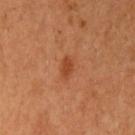No biopsy was performed on this lesion — it was imaged during a full skin examination and was not determined to be concerning. Measured at roughly 2.5 mm in maximum diameter. The lesion-visualizer software estimated a footprint of about 3 mm², an outline eccentricity of about 0.85 (0 = round, 1 = elongated), and a symmetry-axis asymmetry near 0.3. The software also gave a normalized lesion–skin contrast near 7. The software also gave a border-irregularity rating of about 2.5/10 and peripheral color asymmetry of about 0. A female subject in their mid- to late 50s. Located on the arm. A 15 mm close-up tile from a total-body photography series done for melanoma screening.